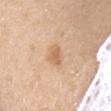biopsy status = imaged on a skin check; not biopsied | TBP lesion metrics = a shape eccentricity near 0.5 and two-axis asymmetry of about 0.25; a lesion color around L≈65 a*≈19 b*≈36 in CIELAB, about 9 CIELAB-L* units darker than the surrounding skin, and a normalized lesion–skin contrast near 6 | acquisition = ~15 mm crop, total-body skin-cancer survey | lighting = white-light | body site = the chest | subject = male, approximately 30 years of age.